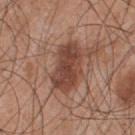| field | value |
|---|---|
| workup | catalogued during a skin exam; not biopsied |
| anatomic site | the chest |
| subject | male, aged 53–57 |
| tile lighting | white-light illumination |
| lesion diameter | about 6 mm |
| image source | ~15 mm crop, total-body skin-cancer survey |